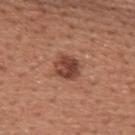This lesion was catalogued during total-body skin photography and was not selected for biopsy. Cropped from a whole-body photographic skin survey; the tile spans about 15 mm. Located on the upper back. A male patient approximately 45 years of age.